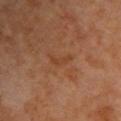Q: Was a biopsy performed?
A: no biopsy performed (imaged during a skin exam)
Q: What lighting was used for the tile?
A: cross-polarized illumination
Q: Where on the body is the lesion?
A: the upper back
Q: What is the imaging modality?
A: ~15 mm crop, total-body skin-cancer survey
Q: What did automated image analysis measure?
A: a mean CIELAB color near L≈41 a*≈22 b*≈34, a lesion–skin lightness drop of about 5, and a normalized border contrast of about 5; border irregularity of about 6.5 on a 0–10 scale, a within-lesion color-variation index near 0/10, and radial color variation of about 0; a classifier nevus-likeness of about 0/100 and a detector confidence of about 100 out of 100 that the crop contains a lesion
Q: Who is the patient?
A: female, aged 63 to 67
Q: What is the lesion's diameter?
A: ≈3 mm